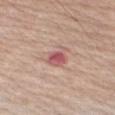follow-up: catalogued during a skin exam; not biopsied
acquisition: 15 mm crop, total-body photography
anatomic site: the right upper arm
subject: male, in their mid- to late 60s
illumination: white-light
lesion diameter: about 3 mm